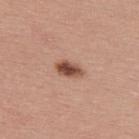patient: male, aged 38–42 | anatomic site: the upper back | acquisition: total-body-photography crop, ~15 mm field of view | lesion size: about 3.5 mm.